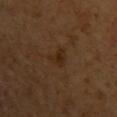Notes:
• notes — total-body-photography surveillance lesion; no biopsy
• imaging modality — total-body-photography crop, ~15 mm field of view
• lighting — cross-polarized
• patient — female, aged approximately 55
• location — the left upper arm
• lesion diameter — ≈3 mm